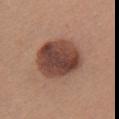Q: Was a biopsy performed?
A: imaged on a skin check; not biopsied
Q: What are the patient's age and sex?
A: female, roughly 35 years of age
Q: What is the anatomic site?
A: the head or neck
Q: What lighting was used for the tile?
A: white-light
Q: Automated lesion metrics?
A: a lesion area of about 24 mm² and a shape-asymmetry score of about 0.1 (0 = symmetric); a detector confidence of about 100 out of 100 that the crop contains a lesion
Q: What is the imaging modality?
A: ~15 mm tile from a whole-body skin photo
Q: How large is the lesion?
A: ~6 mm (longest diameter)